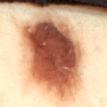A female patient, in their 40s. This image is a 15 mm lesion crop taken from a total-body photograph. Captured under cross-polarized illumination. The lesion is on the upper back. The total-body-photography lesion software estimated an area of roughly 60 mm², a shape eccentricity near 0.85, and a shape-asymmetry score of about 0.15 (0 = symmetric). It also reported a mean CIELAB color near L≈53 a*≈30 b*≈36, roughly 35 lightness units darker than nearby skin, and a normalized border contrast of about 19. The analysis additionally found a color-variation rating of about 10/10 and radial color variation of about 4. It also reported a classifier nevus-likeness of about 100/100 and a detector confidence of about 100 out of 100 that the crop contains a lesion. The lesion's longest dimension is about 13 mm.